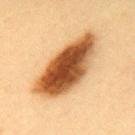biopsy status: imaged on a skin check; not biopsied
body site: the upper back
size: ≈9.5 mm
patient: female, aged approximately 45
tile lighting: cross-polarized illumination
image-analysis metrics: a footprint of about 30 mm², an eccentricity of roughly 0.9, and a shape-asymmetry score of about 0.2 (0 = symmetric); a lesion color around L≈47 a*≈22 b*≈37 in CIELAB, about 22 CIELAB-L* units darker than the surrounding skin, and a lesion-to-skin contrast of about 14.5 (normalized; higher = more distinct)
image: 15 mm crop, total-body photography